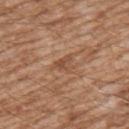From the right upper arm. Automated tile analysis of the lesion measured an average lesion color of about L≈50 a*≈21 b*≈33 (CIELAB). And it measured a within-lesion color-variation index near 3.5/10 and radial color variation of about 1.5. The patient is a male about 60 years old. Measured at roughly 3 mm in maximum diameter. Captured under white-light illumination. Cropped from a total-body skin-imaging series; the visible field is about 15 mm.